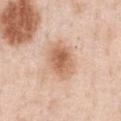Recorded during total-body skin imaging; not selected for excision or biopsy.
On the chest.
A region of skin cropped from a whole-body photographic capture, roughly 15 mm wide.
A male patient aged around 50.
The tile uses white-light illumination.